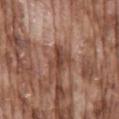Clinical impression: The lesion was tiled from a total-body skin photograph and was not biopsied. Acquisition and patient details: The tile uses white-light illumination. On the mid back. A lesion tile, about 15 mm wide, cut from a 3D total-body photograph. The lesion's longest dimension is about 3 mm. A male subject, roughly 75 years of age.